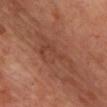biopsy status: no biopsy performed (imaged during a skin exam); subject: aged 63 to 67; anatomic site: the chest; size: ≈7 mm; image: ~15 mm crop, total-body skin-cancer survey.